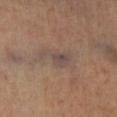Cropped from a total-body skin-imaging series; the visible field is about 15 mm. A female subject aged 68–72. Imaged with cross-polarized lighting. On the right lower leg.